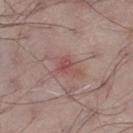Assessment: The lesion was photographed on a routine skin check and not biopsied; there is no pathology result. Clinical summary: A male patient, approximately 70 years of age. The lesion is on the left thigh. A region of skin cropped from a whole-body photographic capture, roughly 15 mm wide.